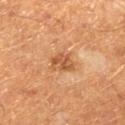Q: Is there a histopathology result?
A: total-body-photography surveillance lesion; no biopsy
Q: Patient demographics?
A: male, aged 58 to 62
Q: Lesion size?
A: ≈3 mm
Q: Automated lesion metrics?
A: a lesion color around L≈50 a*≈23 b*≈37 in CIELAB, roughly 10 lightness units darker than nearby skin, and a normalized lesion–skin contrast near 7; internal color variation of about 3 on a 0–10 scale and peripheral color asymmetry of about 1; a nevus-likeness score of about 15/100 and a lesion-detection confidence of about 100/100
Q: What is the anatomic site?
A: the leg
Q: What lighting was used for the tile?
A: cross-polarized
Q: What kind of image is this?
A: ~15 mm crop, total-body skin-cancer survey